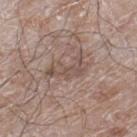* notes: imaged on a skin check; not biopsied
* site: the leg
* TBP lesion metrics: a lesion area of about 8.5 mm², a shape eccentricity near 0.85, and two-axis asymmetry of about 0.45; about 8 CIELAB-L* units darker than the surrounding skin; radial color variation of about 1; a classifier nevus-likeness of about 0/100 and a detector confidence of about 70 out of 100 that the crop contains a lesion
* illumination: white-light illumination
* subject: male, aged around 60
* acquisition: ~15 mm tile from a whole-body skin photo